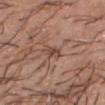Part of a total-body skin-imaging series; this lesion was reviewed on a skin check and was not flagged for biopsy.
A lesion tile, about 15 mm wide, cut from a 3D total-body photograph.
Imaged with white-light lighting.
Located on the head or neck.
A male subject, roughly 40 years of age.